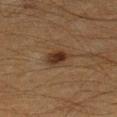The lesion is on the left lower leg. Approximately 3.5 mm at its widest. A roughly 15 mm field-of-view crop from a total-body skin photograph. The subject is a male approximately 50 years of age. The tile uses cross-polarized illumination. Automated image analysis of the tile measured a footprint of about 7.5 mm² and an eccentricity of roughly 0.55. The software also gave a border-irregularity rating of about 2/10 and peripheral color asymmetry of about 1.